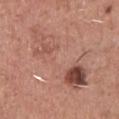Impression: The lesion was tiled from a total-body skin photograph and was not biopsied.